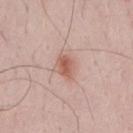Clinical impression:
Captured during whole-body skin photography for melanoma surveillance; the lesion was not biopsied.
Clinical summary:
The lesion is on the mid back. The patient is a male aged around 35. Captured under white-light illumination. A region of skin cropped from a whole-body photographic capture, roughly 15 mm wide.